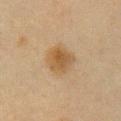Q: Was a biopsy performed?
A: imaged on a skin check; not biopsied
Q: What is the anatomic site?
A: the chest
Q: How was the tile lit?
A: cross-polarized
Q: How large is the lesion?
A: ~3.5 mm (longest diameter)
Q: What is the imaging modality?
A: 15 mm crop, total-body photography
Q: Automated lesion metrics?
A: an average lesion color of about L≈45 a*≈14 b*≈32 (CIELAB), roughly 8 lightness units darker than nearby skin, and a lesion-to-skin contrast of about 7.5 (normalized; higher = more distinct); an automated nevus-likeness rating near 95 out of 100
Q: What are the patient's age and sex?
A: female, in their 40s